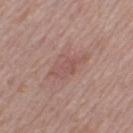Q: Was this lesion biopsied?
A: total-body-photography surveillance lesion; no biopsy
Q: What is the anatomic site?
A: the right thigh
Q: What kind of image is this?
A: 15 mm crop, total-body photography
Q: Illumination type?
A: white-light illumination
Q: What did automated image analysis measure?
A: a lesion area of about 8 mm²; an average lesion color of about L≈53 a*≈21 b*≈23 (CIELAB) and a lesion–skin lightness drop of about 7
Q: Who is the patient?
A: female, aged approximately 40
Q: Lesion size?
A: ≈4.5 mm